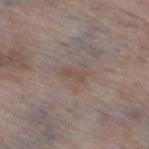Impression:
Recorded during total-body skin imaging; not selected for excision or biopsy.
Context:
On the right thigh. Cropped from a total-body skin-imaging series; the visible field is about 15 mm. A female subject aged 83 or older.